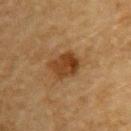<lesion>
  <biopsy_status>not biopsied; imaged during a skin examination</biopsy_status>
  <lesion_size>
    <long_diameter_mm_approx>4.0</long_diameter_mm_approx>
  </lesion_size>
  <image>
    <source>total-body photography crop</source>
    <field_of_view_mm>15</field_of_view_mm>
  </image>
  <site>upper back</site>
  <lighting>cross-polarized</lighting>
  <patient>
    <sex>male</sex>
    <age_approx>85+</age_approx>
  </patient>
</lesion>